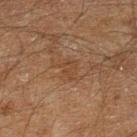Imaged during a routine full-body skin examination; the lesion was not biopsied and no histopathology is available. A 15 mm close-up extracted from a 3D total-body photography capture. Approximately 2.5 mm at its widest. On the left lower leg. Captured under cross-polarized illumination. The lesion-visualizer software estimated an average lesion color of about L≈33 a*≈15 b*≈25 (CIELAB), about 4 CIELAB-L* units darker than the surrounding skin, and a normalized lesion–skin contrast near 4.5. The software also gave border irregularity of about 4 on a 0–10 scale and a color-variation rating of about 1/10. The patient is a male aged approximately 60.